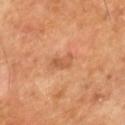Findings:
- biopsy status · catalogued during a skin exam; not biopsied
- image · total-body-photography crop, ~15 mm field of view
- tile lighting · cross-polarized illumination
- subject · male, aged 63 to 67
- lesion size · ~2.5 mm (longest diameter)